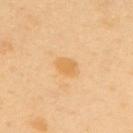The lesion was photographed on a routine skin check and not biopsied; there is no pathology result.
The lesion is located on the mid back.
A 15 mm close-up extracted from a 3D total-body photography capture.
A female subject in their 50s.
Imaged with cross-polarized lighting.
The recorded lesion diameter is about 3 mm.